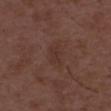biopsy status: catalogued during a skin exam; not biopsied
lesion diameter: about 2.5 mm
image source: total-body-photography crop, ~15 mm field of view
patient: male, in their 50s
tile lighting: white-light illumination
automated metrics: a border-irregularity index near 3.5/10 and peripheral color asymmetry of about 0.5